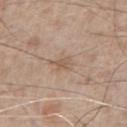Clinical summary: On the front of the torso. The patient is a male roughly 65 years of age. A lesion tile, about 15 mm wide, cut from a 3D total-body photograph.